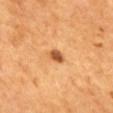Recorded during total-body skin imaging; not selected for excision or biopsy.
On the mid back.
Automated image analysis of the tile measured a lesion area of about 3 mm², an eccentricity of roughly 0.7, and two-axis asymmetry of about 0.2. The analysis additionally found an average lesion color of about L≈56 a*≈27 b*≈43 (CIELAB), roughly 16 lightness units darker than nearby skin, and a normalized lesion–skin contrast near 10. The software also gave a border-irregularity index near 1.5/10, internal color variation of about 2.5 on a 0–10 scale, and radial color variation of about 1.
Cropped from a total-body skin-imaging series; the visible field is about 15 mm.
This is a cross-polarized tile.
A male subject, approximately 65 years of age.